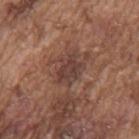Case summary:
• lighting: white-light illumination
• diameter: about 4 mm
• image-analysis metrics: a border-irregularity rating of about 3.5/10, a color-variation rating of about 3/10, and a peripheral color-asymmetry measure near 1; a nevus-likeness score of about 0/100 and lesion-presence confidence of about 90/100
• body site: the back
• imaging modality: ~15 mm crop, total-body skin-cancer survey
• subject: male, in their mid- to late 70s